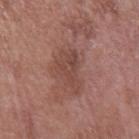Captured during whole-body skin photography for melanoma surveillance; the lesion was not biopsied.
A 15 mm crop from a total-body photograph taken for skin-cancer surveillance.
A male patient, roughly 75 years of age.
Imaged with white-light lighting.
The lesion is located on the arm.